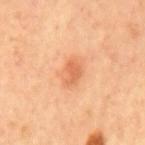Findings:
- notes — no biopsy performed (imaged during a skin exam)
- size — about 3.5 mm
- illumination — cross-polarized
- site — the back
- automated lesion analysis — an area of roughly 5 mm² and a shape eccentricity near 0.8; a color-variation rating of about 2/10; an automated nevus-likeness rating near 95 out of 100 and a lesion-detection confidence of about 100/100
- patient — male, about 65 years old
- image source — ~15 mm crop, total-body skin-cancer survey